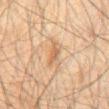{
  "biopsy_status": "not biopsied; imaged during a skin examination",
  "patient": {
    "sex": "male",
    "age_approx": 60
  },
  "site": "chest",
  "image": {
    "source": "total-body photography crop",
    "field_of_view_mm": 15
  },
  "lighting": "cross-polarized"
}